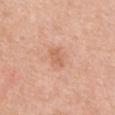| key | value |
|---|---|
| follow-up | imaged on a skin check; not biopsied |
| diameter | ≈2.5 mm |
| lighting | white-light |
| subject | female, in their 40s |
| anatomic site | the front of the torso |
| acquisition | ~15 mm tile from a whole-body skin photo |